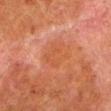workup: catalogued during a skin exam; not biopsied
acquisition: ~15 mm tile from a whole-body skin photo
location: the left lower leg
patient: male, aged 78 to 82